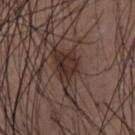• notes · total-body-photography surveillance lesion; no biopsy
• image · 15 mm crop, total-body photography
• patient · male, aged 48 to 52
• lesion diameter · ≈8 mm
• body site · the front of the torso